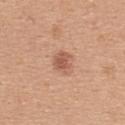{"biopsy_status": "not biopsied; imaged during a skin examination", "lighting": "white-light", "lesion_size": {"long_diameter_mm_approx": 2.5}, "image": {"source": "total-body photography crop", "field_of_view_mm": 15}, "site": "back", "patient": {"sex": "female", "age_approx": 45}}